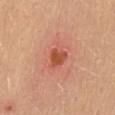The lesion was tiled from a total-body skin photograph and was not biopsied. Imaged with white-light lighting. A female patient aged 43 to 47. The recorded lesion diameter is about 2.5 mm. The lesion is on the mid back. A 15 mm crop from a total-body photograph taken for skin-cancer surveillance.